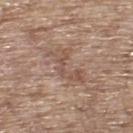<lesion>
  <biopsy_status>not biopsied; imaged during a skin examination</biopsy_status>
  <image>
    <source>total-body photography crop</source>
    <field_of_view_mm>15</field_of_view_mm>
  </image>
  <site>upper back</site>
  <patient>
    <sex>male</sex>
    <age_approx>65</age_approx>
  </patient>
</lesion>